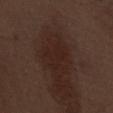Captured during whole-body skin photography for melanoma surveillance; the lesion was not biopsied.
From the abdomen.
A roughly 15 mm field-of-view crop from a total-body skin photograph.
A male subject, aged approximately 70.
The recorded lesion diameter is about 3.5 mm.
The lesion-visualizer software estimated a footprint of about 6.5 mm², an outline eccentricity of about 0.65 (0 = round, 1 = elongated), and a shape-asymmetry score of about 0.4 (0 = symmetric). And it measured a color-variation rating of about 1.5/10 and peripheral color asymmetry of about 0.5. And it measured a classifier nevus-likeness of about 0/100 and a lesion-detection confidence of about 100/100.